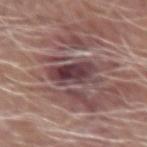notes: catalogued during a skin exam; not biopsied
lighting: white-light illumination
automated metrics: a lesion color around L≈40 a*≈21 b*≈15 in CIELAB and about 14 CIELAB-L* units darker than the surrounding skin; a within-lesion color-variation index near 6/10 and radial color variation of about 2; a nevus-likeness score of about 0/100
image: ~15 mm tile from a whole-body skin photo
site: the right forearm
diameter: ~6 mm (longest diameter)
patient: male, aged around 65